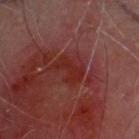follow-up: total-body-photography surveillance lesion; no biopsy | lesion diameter: ~3.5 mm (longest diameter) | subject: male, aged approximately 60 | illumination: cross-polarized illumination | location: the head or neck | automated metrics: an area of roughly 5 mm², an eccentricity of roughly 0.9, and a shape-asymmetry score of about 0.5 (0 = symmetric); a border-irregularity rating of about 6.5/10, internal color variation of about 0.5 on a 0–10 scale, and peripheral color asymmetry of about 0; a nevus-likeness score of about 0/100 and a lesion-detection confidence of about 65/100 | imaging modality: 15 mm crop, total-body photography.